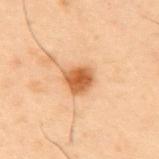Assessment: The lesion was photographed on a routine skin check and not biopsied; there is no pathology result. Acquisition and patient details: The patient is a male in their mid-50s. Longest diameter approximately 3 mm. Located on the mid back. An algorithmic analysis of the crop reported an area of roughly 7 mm² and a shape eccentricity near 0.35. The analysis additionally found an average lesion color of about L≈49 a*≈21 b*≈35 (CIELAB), roughly 13 lightness units darker than nearby skin, and a normalized lesion–skin contrast near 9.5. And it measured a nevus-likeness score of about 100/100 and a lesion-detection confidence of about 100/100. A lesion tile, about 15 mm wide, cut from a 3D total-body photograph.